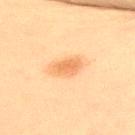Findings:
• biopsy status — no biopsy performed (imaged during a skin exam)
• tile lighting — cross-polarized illumination
• site — the upper back
• image source — 15 mm crop, total-body photography
• subject — male, aged 58–62
• lesion size — ≈3.5 mm